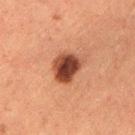Case summary:
- biopsy status · imaged on a skin check; not biopsied
- location · the lower back
- subject · female, about 50 years old
- acquisition · 15 mm crop, total-body photography